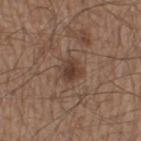<lesion>
  <biopsy_status>not biopsied; imaged during a skin examination</biopsy_status>
  <image>
    <source>total-body photography crop</source>
    <field_of_view_mm>15</field_of_view_mm>
  </image>
  <patient>
    <sex>male</sex>
    <age_approx>60</age_approx>
  </patient>
  <site>left thigh</site>
  <lighting>white-light</lighting>
  <automated_metrics>
    <cielab_L>39</cielab_L>
    <cielab_a>17</cielab_a>
    <cielab_b>26</cielab_b>
    <vs_skin_darker_L>9.0</vs_skin_darker_L>
    <vs_skin_contrast_norm>8.0</vs_skin_contrast_norm>
    <nevus_likeness_0_100>85</nevus_likeness_0_100>
    <lesion_detection_confidence_0_100>100</lesion_detection_confidence_0_100>
  </automated_metrics>
  <lesion_size>
    <long_diameter_mm_approx>2.5</long_diameter_mm_approx>
  </lesion_size>
</lesion>